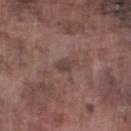This lesion was catalogued during total-body skin photography and was not selected for biopsy. A 15 mm crop from a total-body photograph taken for skin-cancer surveillance. The patient is a male aged 73 to 77. The tile uses white-light illumination. About 2.5 mm across. The lesion is on the left lower leg.